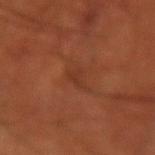Recorded during total-body skin imaging; not selected for excision or biopsy. A close-up tile cropped from a whole-body skin photograph, about 15 mm across. A male patient aged approximately 55. Located on the right forearm. Imaged with cross-polarized lighting. Longest diameter approximately 3.5 mm. An algorithmic analysis of the crop reported an area of roughly 4 mm², an eccentricity of roughly 0.9, and a shape-asymmetry score of about 0.4 (0 = symmetric). It also reported a mean CIELAB color near L≈35 a*≈25 b*≈30 and a normalized border contrast of about 5.